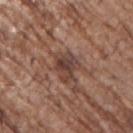Q: Was a biopsy performed?
A: no biopsy performed (imaged during a skin exam)
Q: What is the imaging modality?
A: ~15 mm crop, total-body skin-cancer survey
Q: Lesion location?
A: the front of the torso
Q: Who is the patient?
A: male, roughly 70 years of age